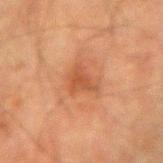Q: Is there a histopathology result?
A: imaged on a skin check; not biopsied